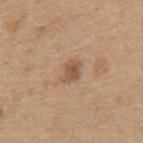Image and clinical context: The patient is a male aged 63–67. The lesion is located on the mid back. Automated image analysis of the tile measured an area of roughly 4.5 mm², an eccentricity of roughly 0.7, and two-axis asymmetry of about 0.25. The analysis additionally found a mean CIELAB color near L≈54 a*≈18 b*≈31 and a lesion-to-skin contrast of about 7 (normalized; higher = more distinct). The software also gave border irregularity of about 2.5 on a 0–10 scale, internal color variation of about 3 on a 0–10 scale, and a peripheral color-asymmetry measure near 1. And it measured an automated nevus-likeness rating near 80 out of 100 and lesion-presence confidence of about 100/100. Imaged with white-light lighting. A roughly 15 mm field-of-view crop from a total-body skin photograph.